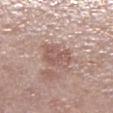biopsy status: total-body-photography surveillance lesion; no biopsy | patient: female, approximately 85 years of age or older | image-analysis metrics: a shape-asymmetry score of about 0.25 (0 = symmetric); a lesion color around L≈56 a*≈20 b*≈23 in CIELAB, a lesion–skin lightness drop of about 9, and a normalized lesion–skin contrast near 6; a border-irregularity index near 2.5/10, a color-variation rating of about 3/10, and a peripheral color-asymmetry measure near 1; a nevus-likeness score of about 0/100 | body site: the leg | image source: 15 mm crop, total-body photography | illumination: white-light illumination.